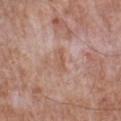Clinical impression:
This lesion was catalogued during total-body skin photography and was not selected for biopsy.
Image and clinical context:
An algorithmic analysis of the crop reported a footprint of about 3.5 mm², an eccentricity of roughly 0.9, and two-axis asymmetry of about 0.25. On the left lower leg. A male subject, roughly 55 years of age. The recorded lesion diameter is about 3 mm. Cropped from a whole-body photographic skin survey; the tile spans about 15 mm. This is a white-light tile.